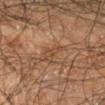The lesion was photographed on a routine skin check and not biopsied; there is no pathology result.
A male patient roughly 55 years of age.
This is a cross-polarized tile.
Automated tile analysis of the lesion measured a lesion area of about 2.5 mm² and a shape eccentricity near 0.95. And it measured a lesion-detection confidence of about 65/100.
Cropped from a whole-body photographic skin survey; the tile spans about 15 mm.
Measured at roughly 3 mm in maximum diameter.
Located on the arm.